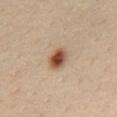<record>
  <biopsy_status>not biopsied; imaged during a skin examination</biopsy_status>
  <patient>
    <sex>male</sex>
    <age_approx>35</age_approx>
  </patient>
  <lighting>cross-polarized</lighting>
  <site>back</site>
  <image>
    <source>total-body photography crop</source>
    <field_of_view_mm>15</field_of_view_mm>
  </image>
  <lesion_size>
    <long_diameter_mm_approx>3.0</long_diameter_mm_approx>
  </lesion_size>
</record>